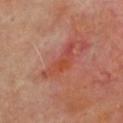<case>
<biopsy_status>not biopsied; imaged during a skin examination</biopsy_status>
</case>